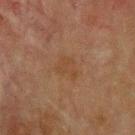Q: Was a biopsy performed?
A: imaged on a skin check; not biopsied
Q: Lesion size?
A: ≈2.5 mm
Q: What are the patient's age and sex?
A: male, about 70 years old
Q: What is the anatomic site?
A: the upper back
Q: How was this image acquired?
A: total-body-photography crop, ~15 mm field of view
Q: Illumination type?
A: cross-polarized illumination
Q: Automated lesion metrics?
A: a footprint of about 4.5 mm², an outline eccentricity of about 0.7 (0 = round, 1 = elongated), and two-axis asymmetry of about 0.4; a nevus-likeness score of about 0/100